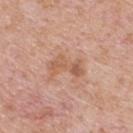Captured during whole-body skin photography for melanoma surveillance; the lesion was not biopsied. A male patient aged 58 to 62. The lesion is located on the upper back. Automated tile analysis of the lesion measured an outline eccentricity of about 0.9 (0 = round, 1 = elongated) and a symmetry-axis asymmetry near 0.4. It also reported border irregularity of about 6.5 on a 0–10 scale, internal color variation of about 4 on a 0–10 scale, and a peripheral color-asymmetry measure near 1.5. It also reported a lesion-detection confidence of about 100/100. Approximately 4 mm at its widest. The tile uses white-light illumination. A 15 mm close-up extracted from a 3D total-body photography capture.